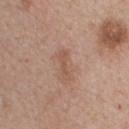Captured during whole-body skin photography for melanoma surveillance; the lesion was not biopsied. The lesion is on the upper back. Imaged with white-light lighting. A 15 mm crop from a total-body photograph taken for skin-cancer surveillance. Longest diameter approximately 3.5 mm. A female subject, aged 43–47.